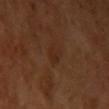  biopsy_status: not biopsied; imaged during a skin examination
  lighting: cross-polarized
  image:
    source: total-body photography crop
    field_of_view_mm: 15
  site: left upper arm
  lesion_size:
    long_diameter_mm_approx: 2.5
  patient:
    sex: female
    age_approx: 55